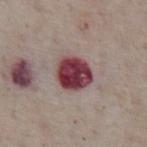Recorded during total-body skin imaging; not selected for excision or biopsy. A lesion tile, about 15 mm wide, cut from a 3D total-body photograph. On the abdomen. The subject is a male aged around 75.